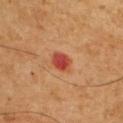Located on the front of the torso. A male subject in their mid-50s. A 15 mm crop from a total-body photograph taken for skin-cancer surveillance.